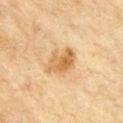Assessment:
The lesion was photographed on a routine skin check and not biopsied; there is no pathology result.